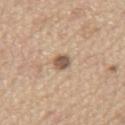Impression:
Imaged during a routine full-body skin examination; the lesion was not biopsied and no histopathology is available.
Image and clinical context:
A male subject approximately 70 years of age. This image is a 15 mm lesion crop taken from a total-body photograph. From the chest.